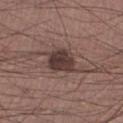notes: total-body-photography surveillance lesion; no biopsy
location: the leg
patient: male, in their mid- to late 30s
lesion diameter: ≈3.5 mm
image-analysis metrics: a detector confidence of about 100 out of 100 that the crop contains a lesion
image source: 15 mm crop, total-body photography
lighting: white-light illumination